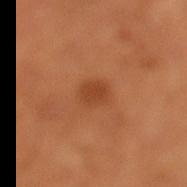Captured during whole-body skin photography for melanoma surveillance; the lesion was not biopsied. The total-body-photography lesion software estimated an area of roughly 3.5 mm², an outline eccentricity of about 0.8 (0 = round, 1 = elongated), and two-axis asymmetry of about 0.25. The software also gave a lesion color around L≈43 a*≈28 b*≈38 in CIELAB and about 8 CIELAB-L* units darker than the surrounding skin. The software also gave a border-irregularity rating of about 2.5/10, a within-lesion color-variation index near 1/10, and peripheral color asymmetry of about 0.5. Cropped from a total-body skin-imaging series; the visible field is about 15 mm. The patient is a male about 50 years old. The lesion is located on the leg. This is a cross-polarized tile.